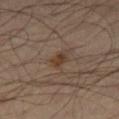Notes:
- follow-up · total-body-photography surveillance lesion; no biopsy
- size · about 2 mm
- subject · male, aged around 45
- image source · total-body-photography crop, ~15 mm field of view
- location · the leg
- illumination · cross-polarized illumination